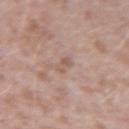biopsy status — no biopsy performed (imaged during a skin exam)
lesion diameter — about 2.5 mm
site — the arm
subject — male, in their 40s
image — ~15 mm tile from a whole-body skin photo
image-analysis metrics — an area of roughly 2.5 mm² and a shape eccentricity near 0.9; about 7 CIELAB-L* units darker than the surrounding skin and a normalized lesion–skin contrast near 5; border irregularity of about 4 on a 0–10 scale, internal color variation of about 0 on a 0–10 scale, and radial color variation of about 0; an automated nevus-likeness rating near 0 out of 100 and a detector confidence of about 100 out of 100 that the crop contains a lesion